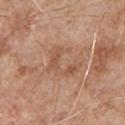Impression: Captured during whole-body skin photography for melanoma surveillance; the lesion was not biopsied. Image and clinical context: This image is a 15 mm lesion crop taken from a total-body photograph. The tile uses white-light illumination. Located on the chest. Approximately 4 mm at its widest. A male subject aged approximately 65. Automated image analysis of the tile measured a nevus-likeness score of about 0/100 and a lesion-detection confidence of about 100/100.